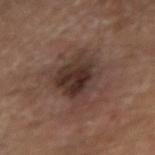A male subject, aged 63 to 67. About 5 mm across. From the right upper arm. A lesion tile, about 15 mm wide, cut from a 3D total-body photograph. The tile uses cross-polarized illumination.